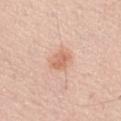Impression:
No biopsy was performed on this lesion — it was imaged during a full skin examination and was not determined to be concerning.
Acquisition and patient details:
A male patient aged approximately 70. Cropped from a whole-body photographic skin survey; the tile spans about 15 mm. The lesion is located on the right thigh.